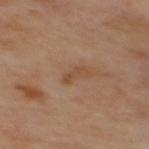Q: Is there a histopathology result?
A: catalogued during a skin exam; not biopsied
Q: How was the tile lit?
A: cross-polarized illumination
Q: What is the imaging modality?
A: total-body-photography crop, ~15 mm field of view
Q: What did automated image analysis measure?
A: a footprint of about 2.5 mm²; a lesion color around L≈48 a*≈20 b*≈32 in CIELAB and a lesion-to-skin contrast of about 6 (normalized; higher = more distinct); a border-irregularity rating of about 5/10, a within-lesion color-variation index near 0/10, and a peripheral color-asymmetry measure near 0; a nevus-likeness score of about 0/100 and lesion-presence confidence of about 100/100
Q: How large is the lesion?
A: ≈3 mm
Q: What is the anatomic site?
A: the mid back